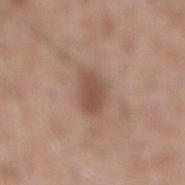Assessment: This lesion was catalogued during total-body skin photography and was not selected for biopsy. Acquisition and patient details: On the mid back. Cropped from a whole-body photographic skin survey; the tile spans about 15 mm. The recorded lesion diameter is about 4 mm. Imaged with white-light lighting. A male patient, in their 60s.